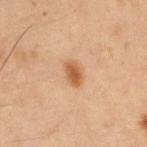Findings:
- biopsy status — total-body-photography surveillance lesion; no biopsy
- location — the right upper arm
- subject — male, aged 48 to 52
- imaging modality — ~15 mm crop, total-body skin-cancer survey